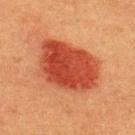Recorded during total-body skin imaging; not selected for excision or biopsy. This is a cross-polarized tile. From the upper back. A male patient aged 38 to 42. A 15 mm close-up tile from a total-body photography series done for melanoma screening. The lesion's longest dimension is about 7.5 mm.